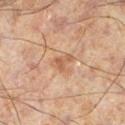follow-up — imaged on a skin check; not biopsied | body site — the left lower leg | imaging modality — total-body-photography crop, ~15 mm field of view | diameter — about 3 mm | subject — male, roughly 60 years of age | automated lesion analysis — a lesion–skin lightness drop of about 7; a classifier nevus-likeness of about 5/100 and a detector confidence of about 100 out of 100 that the crop contains a lesion | illumination — cross-polarized illumination.